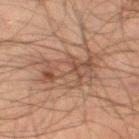Q: Is there a histopathology result?
A: catalogued during a skin exam; not biopsied
Q: What are the patient's age and sex?
A: male, aged approximately 50
Q: Where on the body is the lesion?
A: the left thigh
Q: How was this image acquired?
A: 15 mm crop, total-body photography
Q: How large is the lesion?
A: about 8 mm
Q: How was the tile lit?
A: cross-polarized illumination